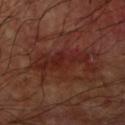Impression: Part of a total-body skin-imaging series; this lesion was reviewed on a skin check and was not flagged for biopsy. Context: The patient is a male roughly 60 years of age. Captured under cross-polarized illumination. A close-up tile cropped from a whole-body skin photograph, about 15 mm across. Automated tile analysis of the lesion measured a lesion color around L≈25 a*≈24 b*≈24 in CIELAB, a lesion–skin lightness drop of about 6, and a lesion-to-skin contrast of about 7 (normalized; higher = more distinct). Located on the left upper arm. The lesion's longest dimension is about 7.5 mm.